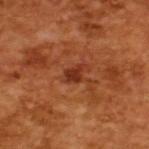Imaged during a routine full-body skin examination; the lesion was not biopsied and no histopathology is available. A roughly 15 mm field-of-view crop from a total-body skin photograph. Imaged with cross-polarized lighting. A male patient aged around 65. Longest diameter approximately 2.5 mm.